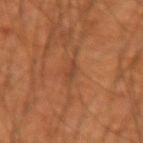| field | value |
|---|---|
| follow-up | no biopsy performed (imaged during a skin exam) |